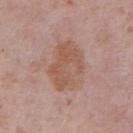workup — imaged on a skin check; not biopsied
imaging modality — ~15 mm tile from a whole-body skin photo
body site — the abdomen
tile lighting — white-light
patient — male, aged 73–77